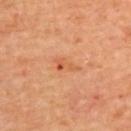The lesion's longest dimension is about 3 mm.
A close-up tile cropped from a whole-body skin photograph, about 15 mm across.
A male patient, in their 70s.
The lesion is on the upper back.
The lesion-visualizer software estimated a lesion area of about 4 mm², a shape eccentricity near 0.85, and two-axis asymmetry of about 0.4. It also reported a mean CIELAB color near L≈57 a*≈27 b*≈39 and a normalized border contrast of about 5.5. It also reported a border-irregularity rating of about 4/10, internal color variation of about 4.5 on a 0–10 scale, and a peripheral color-asymmetry measure near 1.